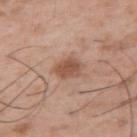notes: no biopsy performed (imaged during a skin exam)
patient: male, in their mid- to late 50s
imaging modality: ~15 mm tile from a whole-body skin photo
TBP lesion metrics: about 11 CIELAB-L* units darker than the surrounding skin and a lesion-to-skin contrast of about 7.5 (normalized; higher = more distinct); a classifier nevus-likeness of about 70/100 and a lesion-detection confidence of about 100/100
location: the arm
diameter: ~3 mm (longest diameter)
illumination: white-light illumination